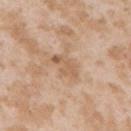No biopsy was performed on this lesion — it was imaged during a full skin examination and was not determined to be concerning.
The patient is a female approximately 25 years of age.
Measured at roughly 3.5 mm in maximum diameter.
A lesion tile, about 15 mm wide, cut from a 3D total-body photograph.
From the right upper arm.